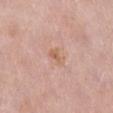This lesion was catalogued during total-body skin photography and was not selected for biopsy. The tile uses white-light illumination. A region of skin cropped from a whole-body photographic capture, roughly 15 mm wide. The patient is a female aged around 40. Located on the right lower leg. An algorithmic analysis of the crop reported an outline eccentricity of about 0.8 (0 = round, 1 = elongated) and a shape-asymmetry score of about 0.3 (0 = symmetric).